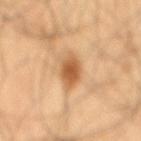Q: Is there a histopathology result?
A: no biopsy performed (imaged during a skin exam)
Q: Lesion location?
A: the back
Q: What is the imaging modality?
A: 15 mm crop, total-body photography
Q: What is the lesion's diameter?
A: ≈3 mm
Q: Patient demographics?
A: male, aged approximately 60
Q: What lighting was used for the tile?
A: cross-polarized